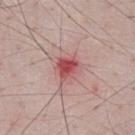{"biopsy_status": "not biopsied; imaged during a skin examination", "site": "front of the torso", "image": {"source": "total-body photography crop", "field_of_view_mm": 15}, "lighting": "white-light", "patient": {"sex": "male", "age_approx": 50}, "lesion_size": {"long_diameter_mm_approx": 3.0}, "automated_metrics": {"nevus_likeness_0_100": 0, "lesion_detection_confidence_0_100": 100}}